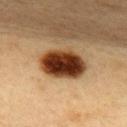Notes:
- follow-up: imaged on a skin check; not biopsied
- location: the upper back
- tile lighting: cross-polarized illumination
- imaging modality: ~15 mm tile from a whole-body skin photo
- subject: female, aged 43 to 47